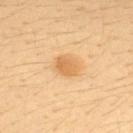Clinical impression: The lesion was photographed on a routine skin check and not biopsied; there is no pathology result. Clinical summary: A 15 mm crop from a total-body photograph taken for skin-cancer surveillance. A female subject, about 35 years old. Measured at roughly 3 mm in maximum diameter. The tile uses cross-polarized illumination. The lesion-visualizer software estimated a within-lesion color-variation index near 3/10. The software also gave an automated nevus-likeness rating near 75 out of 100 and a lesion-detection confidence of about 100/100. The lesion is located on the upper back.